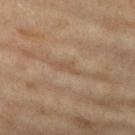Assessment: Recorded during total-body skin imaging; not selected for excision or biopsy. Image and clinical context: The lesion's longest dimension is about 3 mm. Automated image analysis of the tile measured border irregularity of about 4 on a 0–10 scale, a within-lesion color-variation index near 0/10, and peripheral color asymmetry of about 0. The analysis additionally found an automated nevus-likeness rating near 0 out of 100 and a detector confidence of about 55 out of 100 that the crop contains a lesion. A lesion tile, about 15 mm wide, cut from a 3D total-body photograph. Located on the left lower leg. A female patient, roughly 75 years of age.